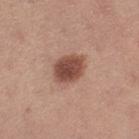The lesion was photographed on a routine skin check and not biopsied; there is no pathology result.
Automated tile analysis of the lesion measured a border-irregularity index near 1.5/10, a color-variation rating of about 4/10, and peripheral color asymmetry of about 1.
A female subject, aged 23 to 27.
From the left thigh.
A 15 mm close-up tile from a total-body photography series done for melanoma screening.
Approximately 4 mm at its widest.
Captured under white-light illumination.